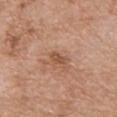Q: What is the imaging modality?
A: 15 mm crop, total-body photography
Q: Illumination type?
A: white-light illumination
Q: Who is the patient?
A: male, about 65 years old
Q: What is the anatomic site?
A: the chest
Q: Lesion size?
A: about 2.5 mm